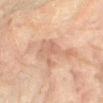- workup: imaged on a skin check; not biopsied
- automated metrics: a border-irregularity index near 10/10 and peripheral color asymmetry of about 0.5
- image source: 15 mm crop, total-body photography
- site: the leg
- patient: female, aged approximately 80
- lighting: cross-polarized
- size: ≈6.5 mm